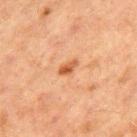<case>
<biopsy_status>not biopsied; imaged during a skin examination</biopsy_status>
<automated_metrics>
  <area_mm2_approx>2.5</area_mm2_approx>
  <eccentricity>0.85</eccentricity>
  <cielab_L>46</cielab_L>
  <cielab_a>23</cielab_a>
  <cielab_b>35</cielab_b>
  <vs_skin_darker_L>10.0</vs_skin_darker_L>
  <vs_skin_contrast_norm>8.5</vs_skin_contrast_norm>
</automated_metrics>
<patient>
  <sex>male</sex>
  <age_approx>65</age_approx>
</patient>
<lesion_size>
  <long_diameter_mm_approx>2.5</long_diameter_mm_approx>
</lesion_size>
<site>front of the torso</site>
<image>
  <source>total-body photography crop</source>
  <field_of_view_mm>15</field_of_view_mm>
</image>
</case>